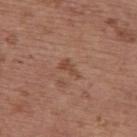  biopsy_status: not biopsied; imaged during a skin examination
  lesion_size:
    long_diameter_mm_approx: 3.0
  patient:
    sex: female
    age_approx: 65
  site: upper back
  image:
    source: total-body photography crop
    field_of_view_mm: 15
  lighting: white-light